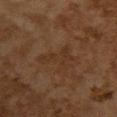No biopsy was performed on this lesion — it was imaged during a full skin examination and was not determined to be concerning. Longest diameter approximately 4.5 mm. A 15 mm close-up extracted from a 3D total-body photography capture. A female patient about 60 years old. Automated tile analysis of the lesion measured a mean CIELAB color near L≈28 a*≈15 b*≈26, a lesion–skin lightness drop of about 4, and a normalized lesion–skin contrast near 4.5. It also reported border irregularity of about 9 on a 0–10 scale, internal color variation of about 1.5 on a 0–10 scale, and a peripheral color-asymmetry measure near 0.5. The lesion is located on the upper back. This is a cross-polarized tile.